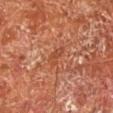The lesion was tiled from a total-body skin photograph and was not biopsied. From the left lower leg. A male subject aged approximately 65. A region of skin cropped from a whole-body photographic capture, roughly 15 mm wide. An algorithmic analysis of the crop reported a lesion area of about 3 mm², an eccentricity of roughly 0.9, and a shape-asymmetry score of about 0.35 (0 = symmetric). And it measured an average lesion color of about L≈46 a*≈28 b*≈34 (CIELAB), about 6 CIELAB-L* units darker than the surrounding skin, and a normalized border contrast of about 5. This is a cross-polarized tile.